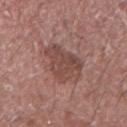Clinical impression: The lesion was tiled from a total-body skin photograph and was not biopsied. Clinical summary: A male patient, aged approximately 70. Longest diameter approximately 4.5 mm. Cropped from a whole-body photographic skin survey; the tile spans about 15 mm. Imaged with white-light lighting. The lesion is on the left forearm.